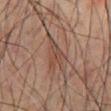notes — no biopsy performed (imaged during a skin exam) | imaging modality — 15 mm crop, total-body photography | location — the mid back | subject — male, roughly 70 years of age.